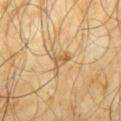biopsy_status: not biopsied; imaged during a skin examination
lighting: cross-polarized
image:
  source: total-body photography crop
  field_of_view_mm: 15
patient:
  sex: male
  age_approx: 65
site: mid back
automated_metrics:
  cielab_L: 57
  cielab_a: 16
  cielab_b: 38
  vs_skin_darker_L: 9.0
  vs_skin_contrast_norm: 6.0
  nevus_likeness_0_100: 0